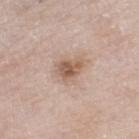Part of a total-body skin-imaging series; this lesion was reviewed on a skin check and was not flagged for biopsy. The lesion is located on the right lower leg. The subject is a male in their 80s. About 3 mm across. A close-up tile cropped from a whole-body skin photograph, about 15 mm across. This is a white-light tile. The total-body-photography lesion software estimated an average lesion color of about L≈57 a*≈18 b*≈28 (CIELAB) and roughly 12 lightness units darker than nearby skin. And it measured a border-irregularity rating of about 2.5/10, a color-variation rating of about 4/10, and a peripheral color-asymmetry measure near 1.5.